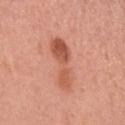Imaged during a routine full-body skin examination; the lesion was not biopsied and no histopathology is available.
The total-body-photography lesion software estimated a lesion area of about 11 mm², an eccentricity of roughly 0.95, and two-axis asymmetry of about 0.35. The analysis additionally found a mean CIELAB color near L≈56 a*≈28 b*≈33. It also reported a border-irregularity index near 5/10, a within-lesion color-variation index near 5.5/10, and a peripheral color-asymmetry measure near 2.
A region of skin cropped from a whole-body photographic capture, roughly 15 mm wide.
On the left upper arm.
A female patient, aged around 50.